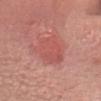No biopsy was performed on this lesion — it was imaged during a full skin examination and was not determined to be concerning.
A 15 mm close-up tile from a total-body photography series done for melanoma screening.
Captured under white-light illumination.
A male subject aged around 45.
The lesion is located on the head or neck.